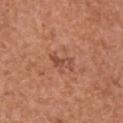This lesion was catalogued during total-body skin photography and was not selected for biopsy. A female subject, approximately 40 years of age. Approximately 3 mm at its widest. From the back. The total-body-photography lesion software estimated an area of roughly 3 mm², an outline eccentricity of about 0.9 (0 = round, 1 = elongated), and a shape-asymmetry score of about 0.4 (0 = symmetric). It also reported an average lesion color of about L≈49 a*≈26 b*≈31 (CIELAB) and a lesion–skin lightness drop of about 8. The software also gave a border-irregularity rating of about 4/10 and a within-lesion color-variation index near 0/10. It also reported a classifier nevus-likeness of about 0/100 and a detector confidence of about 100 out of 100 that the crop contains a lesion. A lesion tile, about 15 mm wide, cut from a 3D total-body photograph.